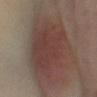The lesion was tiled from a total-body skin photograph and was not biopsied.
A female subject in their mid- to late 50s.
The lesion is on the left lower leg.
This image is a 15 mm lesion crop taken from a total-body photograph.
The recorded lesion diameter is about 7 mm.
Imaged with cross-polarized lighting.